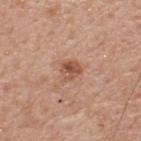Recorded during total-body skin imaging; not selected for excision or biopsy.
A male patient, in their mid-50s.
A lesion tile, about 15 mm wide, cut from a 3D total-body photograph.
Measured at roughly 2.5 mm in maximum diameter.
This is a white-light tile.
Located on the upper back.